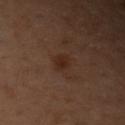This lesion was catalogued during total-body skin photography and was not selected for biopsy.